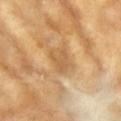The lesion was tiled from a total-body skin photograph and was not biopsied. This is a cross-polarized tile. A region of skin cropped from a whole-body photographic capture, roughly 15 mm wide. The lesion is located on the right upper arm. The total-body-photography lesion software estimated a lesion color around L≈59 a*≈19 b*≈41 in CIELAB and a lesion-to-skin contrast of about 6 (normalized; higher = more distinct). The software also gave a nevus-likeness score of about 0/100. A female subject, aged 73 to 77. Longest diameter approximately 3 mm.